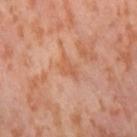Notes:
– notes: total-body-photography surveillance lesion; no biopsy
– image: 15 mm crop, total-body photography
– anatomic site: the right thigh
– patient: female, in their mid-50s